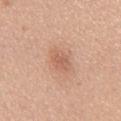<record>
  <image>
    <source>total-body photography crop</source>
    <field_of_view_mm>15</field_of_view_mm>
  </image>
  <patient>
    <sex>female</sex>
    <age_approx>55</age_approx>
  </patient>
  <lighting>white-light</lighting>
  <site>chest</site>
</record>